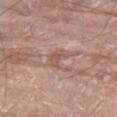Recorded during total-body skin imaging; not selected for excision or biopsy.
A male patient, about 80 years old.
A close-up tile cropped from a whole-body skin photograph, about 15 mm across.
The lesion is located on the leg.